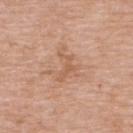Clinical summary:
Located on the upper back. Captured under white-light illumination. A lesion tile, about 15 mm wide, cut from a 3D total-body photograph. A female patient, roughly 65 years of age. Automated tile analysis of the lesion measured a border-irregularity rating of about 9.5/10 and a within-lesion color-variation index near 1/10. The software also gave an automated nevus-likeness rating near 0 out of 100. Measured at roughly 4 mm in maximum diameter.